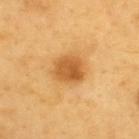  site: upper back
  image:
    source: total-body photography crop
    field_of_view_mm: 15
  lighting: cross-polarized
  patient:
    sex: male
    age_approx: 60